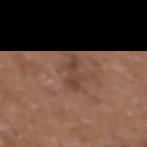Captured during whole-body skin photography for melanoma surveillance; the lesion was not biopsied.